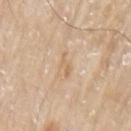follow-up — no biopsy performed (imaged during a skin exam)
illumination — white-light
image source — total-body-photography crop, ~15 mm field of view
diameter — ≈3 mm
automated lesion analysis — a lesion area of about 2.5 mm², an eccentricity of roughly 0.95, and a shape-asymmetry score of about 0.35 (0 = symmetric); roughly 7 lightness units darker than nearby skin and a normalized border contrast of about 5.5; a classifier nevus-likeness of about 0/100 and a detector confidence of about 100 out of 100 that the crop contains a lesion
subject — male, roughly 80 years of age
site — the left upper arm